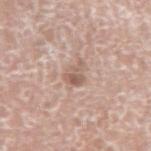No biopsy was performed on this lesion — it was imaged during a full skin examination and was not determined to be concerning. An algorithmic analysis of the crop reported an average lesion color of about L≈58 a*≈18 b*≈25 (CIELAB), about 10 CIELAB-L* units darker than the surrounding skin, and a lesion-to-skin contrast of about 7 (normalized; higher = more distinct). And it measured border irregularity of about 5 on a 0–10 scale and a peripheral color-asymmetry measure near 1. The software also gave a nevus-likeness score of about 0/100 and a lesion-detection confidence of about 100/100. A lesion tile, about 15 mm wide, cut from a 3D total-body photograph. Measured at roughly 2.5 mm in maximum diameter. A male patient, about 75 years old. The lesion is on the right upper arm.